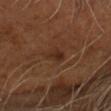The lesion's longest dimension is about 2.5 mm. Cropped from a total-body skin-imaging series; the visible field is about 15 mm. This is a cross-polarized tile. A male patient, in their mid-50s. Located on the head or neck.